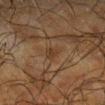The lesion was tiled from a total-body skin photograph and was not biopsied. A male patient in their mid-60s. Automated image analysis of the tile measured a within-lesion color-variation index near 0.5/10. The analysis additionally found a nevus-likeness score of about 0/100 and a detector confidence of about 90 out of 100 that the crop contains a lesion. The recorded lesion diameter is about 2.5 mm. The tile uses cross-polarized illumination. A 15 mm close-up tile from a total-body photography series done for melanoma screening. On the leg.